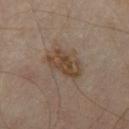<lesion>
  <biopsy_status>not biopsied; imaged during a skin examination</biopsy_status>
  <lesion_size>
    <long_diameter_mm_approx>4.5</long_diameter_mm_approx>
  </lesion_size>
  <patient>
    <age_approx>55</age_approx>
  </patient>
  <site>right thigh</site>
  <image>
    <source>total-body photography crop</source>
    <field_of_view_mm>15</field_of_view_mm>
  </image>
  <lighting>cross-polarized</lighting>
</lesion>